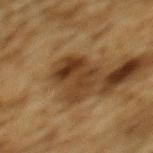The lesion was tiled from a total-body skin photograph and was not biopsied.